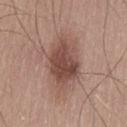Impression: Imaged during a routine full-body skin examination; the lesion was not biopsied and no histopathology is available. Context: The subject is a male aged approximately 55. Automated tile analysis of the lesion measured a footprint of about 20 mm², an outline eccentricity of about 0.8 (0 = round, 1 = elongated), and a shape-asymmetry score of about 0.2 (0 = symmetric). The software also gave border irregularity of about 2.5 on a 0–10 scale and peripheral color asymmetry of about 1.5. The analysis additionally found a nevus-likeness score of about 85/100 and lesion-presence confidence of about 100/100. Captured under white-light illumination. The lesion is located on the left thigh. Approximately 6.5 mm at its widest. A close-up tile cropped from a whole-body skin photograph, about 15 mm across.